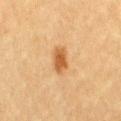This lesion was catalogued during total-body skin photography and was not selected for biopsy.
The lesion is on the right thigh.
The lesion's longest dimension is about 3 mm.
Captured under cross-polarized illumination.
Cropped from a whole-body photographic skin survey; the tile spans about 15 mm.
Automated image analysis of the tile measured an eccentricity of roughly 0.75 and a symmetry-axis asymmetry near 0.25. It also reported a lesion color around L≈49 a*≈21 b*≈38 in CIELAB. And it measured an automated nevus-likeness rating near 95 out of 100 and a lesion-detection confidence of about 100/100.
A female subject, aged approximately 55.